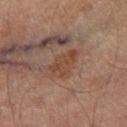workup: catalogued during a skin exam; not biopsied | imaging modality: total-body-photography crop, ~15 mm field of view | subject: male, aged 68–72 | automated metrics: an outline eccentricity of about 0.85 (0 = round, 1 = elongated) and two-axis asymmetry of about 0.3; a nevus-likeness score of about 5/100 and a detector confidence of about 100 out of 100 that the crop contains a lesion | diameter: about 4.5 mm | body site: the right thigh | tile lighting: cross-polarized illumination.